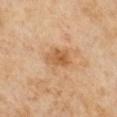Impression:
The lesion was photographed on a routine skin check and not biopsied; there is no pathology result.
Image and clinical context:
A male patient aged around 65. This is a cross-polarized tile. The lesion-visualizer software estimated a lesion area of about 6 mm², a shape eccentricity near 0.75, and a shape-asymmetry score of about 0.2 (0 = symmetric). And it measured a border-irregularity rating of about 2.5/10, a color-variation rating of about 4/10, and peripheral color asymmetry of about 1.5. The software also gave lesion-presence confidence of about 100/100. The lesion's longest dimension is about 3.5 mm. A 15 mm close-up tile from a total-body photography series done for melanoma screening.